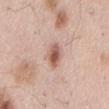No biopsy was performed on this lesion — it was imaged during a full skin examination and was not determined to be concerning. The patient is a male roughly 55 years of age. From the back. Approximately 3.5 mm at its widest. This is a white-light tile. A lesion tile, about 15 mm wide, cut from a 3D total-body photograph.